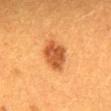{
  "site": "back",
  "automated_metrics": {
    "area_mm2_approx": 10.0,
    "eccentricity": 0.55,
    "shape_asymmetry": 0.15,
    "cielab_L": 46,
    "cielab_a": 25,
    "cielab_b": 38,
    "vs_skin_darker_L": 12.0,
    "nevus_likeness_0_100": 100,
    "lesion_detection_confidence_0_100": 100
  },
  "lesion_size": {
    "long_diameter_mm_approx": 4.0
  },
  "image": {
    "source": "total-body photography crop",
    "field_of_view_mm": 15
  },
  "lighting": "cross-polarized",
  "patient": {
    "sex": "female",
    "age_approx": 40
  }
}